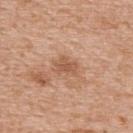Assessment: No biopsy was performed on this lesion — it was imaged during a full skin examination and was not determined to be concerning. Background: Cropped from a whole-body photographic skin survey; the tile spans about 15 mm. From the upper back. Imaged with white-light lighting. The total-body-photography lesion software estimated a lesion–skin lightness drop of about 9 and a normalized lesion–skin contrast near 6.5. The analysis additionally found a classifier nevus-likeness of about 0/100 and a detector confidence of about 100 out of 100 that the crop contains a lesion. A male subject approximately 65 years of age.